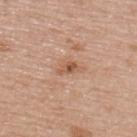The lesion was photographed on a routine skin check and not biopsied; there is no pathology result.